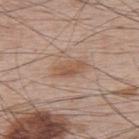follow-up=total-body-photography surveillance lesion; no biopsy | patient=male, aged around 65 | acquisition=15 mm crop, total-body photography | site=the upper back.